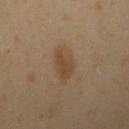Assessment:
This lesion was catalogued during total-body skin photography and was not selected for biopsy.
Image and clinical context:
Automated image analysis of the tile measured an area of roughly 8 mm² and two-axis asymmetry of about 0.15. And it measured a lesion color around L≈45 a*≈15 b*≈31 in CIELAB and a lesion-to-skin contrast of about 6.5 (normalized; higher = more distinct). The software also gave a border-irregularity index near 2/10 and internal color variation of about 2.5 on a 0–10 scale. A male subject aged 38 to 42. Cropped from a whole-body photographic skin survey; the tile spans about 15 mm. From the right upper arm. About 4 mm across. This is a cross-polarized tile.